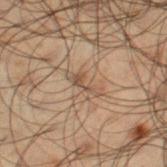No biopsy was performed on this lesion — it was imaged during a full skin examination and was not determined to be concerning.
On the leg.
Captured under cross-polarized illumination.
The total-body-photography lesion software estimated a nevus-likeness score of about 0/100 and a detector confidence of about 50 out of 100 that the crop contains a lesion.
Approximately 3.5 mm at its widest.
A 15 mm close-up extracted from a 3D total-body photography capture.
A male subject aged around 50.